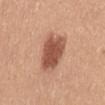Part of a total-body skin-imaging series; this lesion was reviewed on a skin check and was not flagged for biopsy. Longest diameter approximately 6 mm. This is a white-light tile. A 15 mm close-up tile from a total-body photography series done for melanoma screening. The lesion is located on the abdomen. A female patient, in their mid-50s.